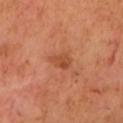Part of a total-body skin-imaging series; this lesion was reviewed on a skin check and was not flagged for biopsy. Automated image analysis of the tile measured an area of roughly 4 mm², a shape eccentricity near 0.85, and a symmetry-axis asymmetry near 0.2. It also reported a mean CIELAB color near L≈51 a*≈29 b*≈38, roughly 9 lightness units darker than nearby skin, and a normalized lesion–skin contrast near 6.5. And it measured a within-lesion color-variation index near 3/10 and a peripheral color-asymmetry measure near 1. It also reported a lesion-detection confidence of about 100/100. This is a cross-polarized tile. A roughly 15 mm field-of-view crop from a total-body skin photograph. About 3 mm across. From the head or neck.